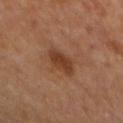Q: Is there a histopathology result?
A: no biopsy performed (imaged during a skin exam)
Q: Lesion size?
A: ~4 mm (longest diameter)
Q: Illumination type?
A: cross-polarized illumination
Q: What did automated image analysis measure?
A: a border-irregularity rating of about 2/10 and a color-variation rating of about 2.5/10; a nevus-likeness score of about 70/100 and lesion-presence confidence of about 100/100
Q: Where on the body is the lesion?
A: the mid back
Q: What kind of image is this?
A: ~15 mm tile from a whole-body skin photo
Q: What are the patient's age and sex?
A: male, in their mid-60s